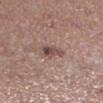Imaged during a routine full-body skin examination; the lesion was not biopsied and no histopathology is available. Cropped from a total-body skin-imaging series; the visible field is about 15 mm. The lesion is on the left lower leg. Imaged with white-light lighting. The total-body-photography lesion software estimated an outline eccentricity of about 0.75 (0 = round, 1 = elongated) and a symmetry-axis asymmetry near 0.35. It also reported an average lesion color of about L≈47 a*≈18 b*≈20 (CIELAB), roughly 11 lightness units darker than nearby skin, and a lesion-to-skin contrast of about 8 (normalized; higher = more distinct). The analysis additionally found border irregularity of about 3 on a 0–10 scale, a within-lesion color-variation index near 3.5/10, and radial color variation of about 1. The software also gave a nevus-likeness score of about 10/100 and a detector confidence of about 100 out of 100 that the crop contains a lesion. The patient is a female aged approximately 60.